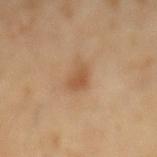Impression:
Part of a total-body skin-imaging series; this lesion was reviewed on a skin check and was not flagged for biopsy.
Image and clinical context:
The lesion is located on the back. This image is a 15 mm lesion crop taken from a total-body photograph. A male subject, aged around 55. About 3 mm across.